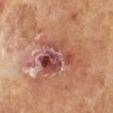Impression: No biopsy was performed on this lesion — it was imaged during a full skin examination and was not determined to be concerning. Context: Imaged with cross-polarized lighting. Longest diameter approximately 6 mm. Cropped from a whole-body photographic skin survey; the tile spans about 15 mm. The patient is a female about 65 years old. The lesion-visualizer software estimated an area of roughly 16 mm², an outline eccentricity of about 0.6 (0 = round, 1 = elongated), and a shape-asymmetry score of about 0.4 (0 = symmetric). And it measured a lesion color around L≈47 a*≈27 b*≈26 in CIELAB, roughly 10 lightness units darker than nearby skin, and a normalized border contrast of about 8.5. And it measured border irregularity of about 6 on a 0–10 scale, a within-lesion color-variation index near 10/10, and a peripheral color-asymmetry measure near 4. And it measured an automated nevus-likeness rating near 0 out of 100 and a detector confidence of about 100 out of 100 that the crop contains a lesion. Located on the right lower leg.